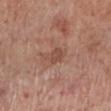Q: Was a biopsy performed?
A: catalogued during a skin exam; not biopsied
Q: Lesion location?
A: the left lower leg
Q: Who is the patient?
A: male, approximately 70 years of age
Q: What lighting was used for the tile?
A: white-light
Q: What is the imaging modality?
A: total-body-photography crop, ~15 mm field of view
Q: Lesion size?
A: ≈3 mm